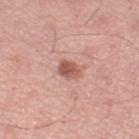biopsy status: catalogued during a skin exam; not biopsied
lesion diameter: ~3 mm (longest diameter)
location: the left thigh
patient: male, aged around 70
lighting: white-light illumination
acquisition: ~15 mm tile from a whole-body skin photo
automated metrics: a footprint of about 5 mm², an outline eccentricity of about 0.65 (0 = round, 1 = elongated), and a symmetry-axis asymmetry near 0.2; a mean CIELAB color near L≈57 a*≈25 b*≈27, about 12 CIELAB-L* units darker than the surrounding skin, and a normalized lesion–skin contrast near 8; border irregularity of about 2 on a 0–10 scale, a within-lesion color-variation index near 3.5/10, and radial color variation of about 1; a classifier nevus-likeness of about 90/100